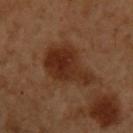{
  "biopsy_status": "not biopsied; imaged during a skin examination",
  "site": "left upper arm",
  "lighting": "cross-polarized",
  "automated_metrics": {
    "lesion_detection_confidence_0_100": 100
  },
  "patient": {
    "sex": "male",
    "age_approx": 50
  },
  "image": {
    "source": "total-body photography crop",
    "field_of_view_mm": 15
  }
}